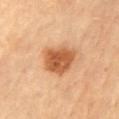| feature | finding |
|---|---|
| patient | male, roughly 85 years of age or older |
| anatomic site | the left upper arm |
| acquisition | 15 mm crop, total-body photography |